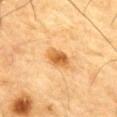{"biopsy_status": "not biopsied; imaged during a skin examination", "image": {"source": "total-body photography crop", "field_of_view_mm": 15}, "patient": {"sex": "male", "age_approx": "85+"}, "automated_metrics": {"area_mm2_approx": 6.0, "eccentricity": 0.75, "shape_asymmetry": 0.25}, "site": "front of the torso", "lesion_size": {"long_diameter_mm_approx": 3.5}}